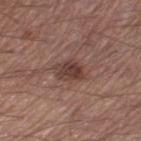Impression:
The lesion was photographed on a routine skin check and not biopsied; there is no pathology result.
Image and clinical context:
Approximately 3.5 mm at its widest. A male patient in their mid-50s. The total-body-photography lesion software estimated a mean CIELAB color near L≈40 a*≈19 b*≈23, roughly 10 lightness units darker than nearby skin, and a normalized border contrast of about 8.5. And it measured border irregularity of about 2 on a 0–10 scale, a color-variation rating of about 4.5/10, and a peripheral color-asymmetry measure near 1.5. The software also gave a nevus-likeness score of about 80/100. A close-up tile cropped from a whole-body skin photograph, about 15 mm across. Captured under white-light illumination. The lesion is on the right thigh.